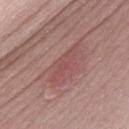Q: Was a biopsy performed?
A: imaged on a skin check; not biopsied
Q: What is the imaging modality?
A: 15 mm crop, total-body photography
Q: What is the anatomic site?
A: the front of the torso
Q: Illumination type?
A: white-light illumination
Q: What is the lesion's diameter?
A: ≈5 mm
Q: What are the patient's age and sex?
A: male, roughly 70 years of age
Q: Automated lesion metrics?
A: an area of roughly 6.5 mm² and a symmetry-axis asymmetry near 0.25; a color-variation rating of about 2/10 and peripheral color asymmetry of about 0.5